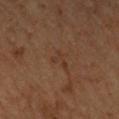This lesion was catalogued during total-body skin photography and was not selected for biopsy. A region of skin cropped from a whole-body photographic capture, roughly 15 mm wide. The total-body-photography lesion software estimated a footprint of about 3 mm², an outline eccentricity of about 0.8 (0 = round, 1 = elongated), and a symmetry-axis asymmetry near 0.6. It also reported a mean CIELAB color near L≈28 a*≈15 b*≈23, about 4 CIELAB-L* units darker than the surrounding skin, and a normalized border contrast of about 4.5. The analysis additionally found a border-irregularity index near 8/10, internal color variation of about 0 on a 0–10 scale, and peripheral color asymmetry of about 0. The lesion is located on the chest. The patient is a male aged 53 to 57. Measured at roughly 3 mm in maximum diameter. Captured under cross-polarized illumination.